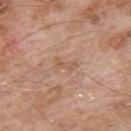Clinical impression:
Recorded during total-body skin imaging; not selected for excision or biopsy.
Acquisition and patient details:
This image is a 15 mm lesion crop taken from a total-body photograph. A male subject, aged approximately 55. About 2.5 mm across. The lesion-visualizer software estimated a footprint of about 3 mm², an outline eccentricity of about 0.9 (0 = round, 1 = elongated), and a shape-asymmetry score of about 0.4 (0 = symmetric). Imaged with white-light lighting. From the upper back.